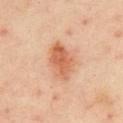| feature | finding |
|---|---|
| notes | catalogued during a skin exam; not biopsied |
| diameter | ≈5 mm |
| automated lesion analysis | a footprint of about 11 mm²; a within-lesion color-variation index near 5.5/10 and peripheral color asymmetry of about 2 |
| illumination | cross-polarized illumination |
| patient | male, about 50 years old |
| anatomic site | the chest |
| acquisition | total-body-photography crop, ~15 mm field of view |